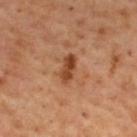  biopsy_status: not biopsied; imaged during a skin examination
  automated_metrics:
    cielab_L: 43
    cielab_a: 24
    cielab_b: 35
    vs_skin_darker_L: 12.0
    vs_skin_contrast_norm: 9.5
    lesion_detection_confidence_0_100: 100
  image:
    source: total-body photography crop
    field_of_view_mm: 15
  patient:
    sex: male
    age_approx: 55
  site: upper back
  lighting: cross-polarized